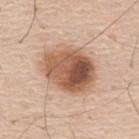biopsy status=catalogued during a skin exam; not biopsied
acquisition=15 mm crop, total-body photography
patient=male, about 60 years old
lesion size=~6.5 mm (longest diameter)
body site=the upper back
lighting=white-light